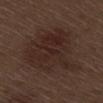follow-up = no biopsy performed (imaged during a skin exam).